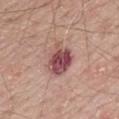Impression:
Part of a total-body skin-imaging series; this lesion was reviewed on a skin check and was not flagged for biopsy.
Acquisition and patient details:
From the mid back. A region of skin cropped from a whole-body photographic capture, roughly 15 mm wide. The subject is a male roughly 70 years of age.